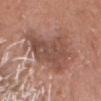Imaged during a routine full-body skin examination; the lesion was not biopsied and no histopathology is available.
A region of skin cropped from a whole-body photographic capture, roughly 15 mm wide.
From the head or neck.
A male subject, about 55 years old.